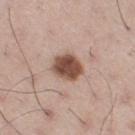- biopsy status · total-body-photography surveillance lesion; no biopsy
- subject · male, in their mid- to late 50s
- lesion size · about 3.5 mm
- body site · the leg
- illumination · white-light illumination
- acquisition · ~15 mm crop, total-body skin-cancer survey